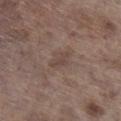The lesion was tiled from a total-body skin photograph and was not biopsied. A male subject, aged 68–72. Cropped from a total-body skin-imaging series; the visible field is about 15 mm. Imaged with white-light lighting. On the right lower leg. Measured at roughly 3 mm in maximum diameter.